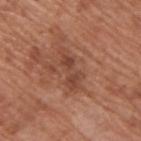Captured during whole-body skin photography for melanoma surveillance; the lesion was not biopsied. On the upper back. Cropped from a total-body skin-imaging series; the visible field is about 15 mm. A male patient, aged approximately 65.